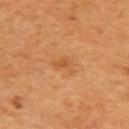follow-up = no biopsy performed (imaged during a skin exam)
body site = the right upper arm
image = total-body-photography crop, ~15 mm field of view
lighting = cross-polarized illumination
subject = male, roughly 55 years of age
size = ~2.5 mm (longest diameter)
TBP lesion metrics = a lesion color around L≈51 a*≈23 b*≈39 in CIELAB, a lesion–skin lightness drop of about 6, and a normalized border contrast of about 4.5; an automated nevus-likeness rating near 0 out of 100 and lesion-presence confidence of about 100/100